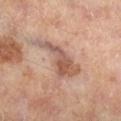Part of a total-body skin-imaging series; this lesion was reviewed on a skin check and was not flagged for biopsy.
Longest diameter approximately 7 mm.
This image is a 15 mm lesion crop taken from a total-body photograph.
The patient is a male roughly 65 years of age.
The lesion is located on the right lower leg.